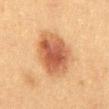| feature | finding |
|---|---|
| follow-up | no biopsy performed (imaged during a skin exam) |
| diameter | ≈6 mm |
| patient | male, roughly 50 years of age |
| imaging modality | ~15 mm crop, total-body skin-cancer survey |
| site | the abdomen |
| lighting | cross-polarized illumination |
| automated lesion analysis | a footprint of about 22 mm² and two-axis asymmetry of about 0.15; a border-irregularity index near 1.5/10, internal color variation of about 6 on a 0–10 scale, and peripheral color asymmetry of about 1.5 |